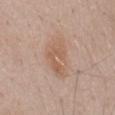Captured during whole-body skin photography for melanoma surveillance; the lesion was not biopsied.
A male subject, aged around 55.
Captured under white-light illumination.
Approximately 5.5 mm at its widest.
Cropped from a whole-body photographic skin survey; the tile spans about 15 mm.
The lesion is located on the mid back.